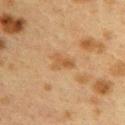| field | value |
|---|---|
| follow-up | catalogued during a skin exam; not biopsied |
| image-analysis metrics | an area of roughly 4.5 mm², an outline eccentricity of about 0.75 (0 = round, 1 = elongated), and a shape-asymmetry score of about 0.45 (0 = symmetric); an average lesion color of about L≈46 a*≈17 b*≈34 (CIELAB), roughly 7 lightness units darker than nearby skin, and a normalized border contrast of about 6; border irregularity of about 4.5 on a 0–10 scale, internal color variation of about 2.5 on a 0–10 scale, and peripheral color asymmetry of about 1; an automated nevus-likeness rating near 0 out of 100 and a detector confidence of about 100 out of 100 that the crop contains a lesion |
| site | the upper back |
| lighting | cross-polarized |
| image source | total-body-photography crop, ~15 mm field of view |
| subject | female, aged around 40 |
| diameter | about 2.5 mm |